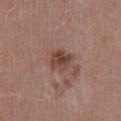Q: Was a biopsy performed?
A: no biopsy performed (imaged during a skin exam)